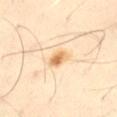Q: Was a biopsy performed?
A: catalogued during a skin exam; not biopsied
Q: How was this image acquired?
A: ~15 mm crop, total-body skin-cancer survey
Q: How large is the lesion?
A: about 2.5 mm
Q: Patient demographics?
A: male, about 30 years old
Q: What lighting was used for the tile?
A: cross-polarized illumination
Q: Where on the body is the lesion?
A: the right thigh